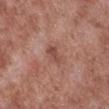Assessment: Imaged during a routine full-body skin examination; the lesion was not biopsied and no histopathology is available. Context: A male subject aged 53 to 57. Cropped from a whole-body photographic skin survey; the tile spans about 15 mm. Imaged with white-light lighting. Longest diameter approximately 2.5 mm. Located on the right lower leg. An algorithmic analysis of the crop reported a peripheral color-asymmetry measure near 0.5. And it measured a detector confidence of about 100 out of 100 that the crop contains a lesion.